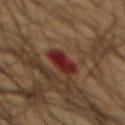Case summary:
* notes: imaged on a skin check; not biopsied
* illumination: cross-polarized
* site: the abdomen
* imaging modality: ~15 mm crop, total-body skin-cancer survey
* lesion diameter: about 3 mm
* patient: male, roughly 65 years of age
* image-analysis metrics: border irregularity of about 2 on a 0–10 scale, a within-lesion color-variation index near 2.5/10, and radial color variation of about 1; a classifier nevus-likeness of about 0/100 and lesion-presence confidence of about 100/100